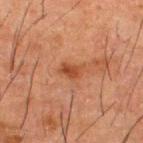<lesion>
<biopsy_status>not biopsied; imaged during a skin examination</biopsy_status>
<site>upper back</site>
<lighting>cross-polarized</lighting>
<image>
  <source>total-body photography crop</source>
  <field_of_view_mm>15</field_of_view_mm>
</image>
<lesion_size>
  <long_diameter_mm_approx>2.5</long_diameter_mm_approx>
</lesion_size>
<patient>
  <sex>male</sex>
  <age_approx>50</age_approx>
</patient>
<automated_metrics>
  <area_mm2_approx>4.0</area_mm2_approx>
  <eccentricity>0.8</eccentricity>
  <shape_asymmetry>0.2</shape_asymmetry>
  <cielab_L>37</cielab_L>
  <cielab_a>23</cielab_a>
  <cielab_b>30</cielab_b>
  <vs_skin_darker_L>8.0</vs_skin_darker_L>
  <vs_skin_contrast_norm>7.5</vs_skin_contrast_norm>
  <border_irregularity_0_10>2.0</border_irregularity_0_10>
  <color_variation_0_10>2.5</color_variation_0_10>
  <nevus_likeness_0_100>65</nevus_likeness_0_100>
</automated_metrics>
</lesion>